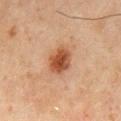Clinical impression:
The lesion was photographed on a routine skin check and not biopsied; there is no pathology result.
Context:
A male patient in their mid-40s. A 15 mm crop from a total-body photograph taken for skin-cancer surveillance. On the mid back. This is a cross-polarized tile. About 3.5 mm across. An algorithmic analysis of the crop reported an outline eccentricity of about 0.6 (0 = round, 1 = elongated) and a symmetry-axis asymmetry near 0.15. It also reported a mean CIELAB color near L≈41 a*≈21 b*≈30 and a lesion-to-skin contrast of about 10 (normalized; higher = more distinct). The software also gave border irregularity of about 1.5 on a 0–10 scale and radial color variation of about 1. The analysis additionally found an automated nevus-likeness rating near 100 out of 100 and a detector confidence of about 100 out of 100 that the crop contains a lesion.